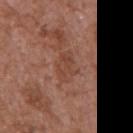Image and clinical context: A 15 mm close-up tile from a total-body photography series done for melanoma screening. A male patient in their mid- to late 70s. From the chest. Automated image analysis of the tile measured an outline eccentricity of about 0.7 (0 = round, 1 = elongated) and a shape-asymmetry score of about 0.3 (0 = symmetric). The software also gave about 6 CIELAB-L* units darker than the surrounding skin. It also reported a lesion-detection confidence of about 100/100. About 3 mm across. The tile uses white-light illumination.